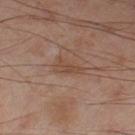Q: Was this lesion biopsied?
A: imaged on a skin check; not biopsied
Q: What lighting was used for the tile?
A: cross-polarized
Q: Automated lesion metrics?
A: a footprint of about 4 mm², an outline eccentricity of about 0.85 (0 = round, 1 = elongated), and two-axis asymmetry of about 0.5; roughly 6 lightness units darker than nearby skin and a lesion-to-skin contrast of about 6 (normalized; higher = more distinct); border irregularity of about 5 on a 0–10 scale and a within-lesion color-variation index near 1.5/10; an automated nevus-likeness rating near 0 out of 100 and lesion-presence confidence of about 100/100
Q: Where on the body is the lesion?
A: the left thigh
Q: Patient demographics?
A: male, aged approximately 55
Q: How was this image acquired?
A: total-body-photography crop, ~15 mm field of view
Q: Lesion size?
A: ≈3 mm